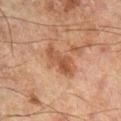Impression:
The lesion was photographed on a routine skin check and not biopsied; there is no pathology result.
Clinical summary:
Cropped from a total-body skin-imaging series; the visible field is about 15 mm. Captured under cross-polarized illumination. The recorded lesion diameter is about 4.5 mm. From the left leg. The subject is a male roughly 60 years of age.